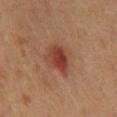Captured during whole-body skin photography for melanoma surveillance; the lesion was not biopsied.
About 4 mm across.
This is a cross-polarized tile.
A male subject, roughly 60 years of age.
On the chest.
A close-up tile cropped from a whole-body skin photograph, about 15 mm across.